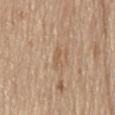Assessment: This lesion was catalogued during total-body skin photography and was not selected for biopsy. Clinical summary: A male patient, roughly 70 years of age. A 15 mm crop from a total-body photograph taken for skin-cancer surveillance. About 2.5 mm across. Automated tile analysis of the lesion measured an area of roughly 2 mm², an eccentricity of roughly 0.95, and a symmetry-axis asymmetry near 0.25. It also reported about 7 CIELAB-L* units darker than the surrounding skin. The analysis additionally found a border-irregularity index near 3/10, a within-lesion color-variation index near 0/10, and radial color variation of about 0. The tile uses white-light illumination. Located on the lower back.